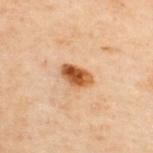Assessment: This lesion was catalogued during total-body skin photography and was not selected for biopsy. Context: A 15 mm close-up extracted from a 3D total-body photography capture. On the upper back. An algorithmic analysis of the crop reported an outline eccentricity of about 0.8 (0 = round, 1 = elongated) and two-axis asymmetry of about 0.2. The analysis additionally found a border-irregularity rating of about 2/10, internal color variation of about 6.5 on a 0–10 scale, and peripheral color asymmetry of about 2.5. And it measured an automated nevus-likeness rating near 100 out of 100 and lesion-presence confidence of about 100/100. Longest diameter approximately 3.5 mm. Imaged with cross-polarized lighting. The subject is a male aged 48 to 52.